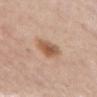Background: Measured at roughly 3.5 mm in maximum diameter. A female subject in their 60s. The lesion is located on the chest. Automated image analysis of the tile measured an area of roughly 7.5 mm², an outline eccentricity of about 0.7 (0 = round, 1 = elongated), and two-axis asymmetry of about 0.25. It also reported a border-irregularity index near 2/10 and a peripheral color-asymmetry measure near 1.5. Captured under white-light illumination. A 15 mm crop from a total-body photograph taken for skin-cancer surveillance.